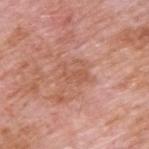Assessment: Recorded during total-body skin imaging; not selected for excision or biopsy. Acquisition and patient details: The subject is a male approximately 60 years of age. The lesion's longest dimension is about 3.5 mm. The lesion is on the upper back. A lesion tile, about 15 mm wide, cut from a 3D total-body photograph. The lesion-visualizer software estimated a lesion area of about 4 mm², an outline eccentricity of about 0.85 (0 = round, 1 = elongated), and a shape-asymmetry score of about 0.6 (0 = symmetric). The software also gave internal color variation of about 0.5 on a 0–10 scale and a peripheral color-asymmetry measure near 0. Captured under white-light illumination.